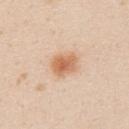{"biopsy_status": "not biopsied; imaged during a skin examination", "patient": {"sex": "male", "age_approx": 25}, "lighting": "white-light", "automated_metrics": {"area_mm2_approx": 7.5, "eccentricity": 0.6, "shape_asymmetry": 0.15}, "site": "upper back", "image": {"source": "total-body photography crop", "field_of_view_mm": 15}, "lesion_size": {"long_diameter_mm_approx": 3.0}}